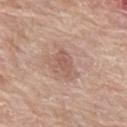| key | value |
|---|---|
| notes | catalogued during a skin exam; not biopsied |
| patient | female, aged approximately 60 |
| lesion diameter | about 3.5 mm |
| automated lesion analysis | a symmetry-axis asymmetry near 0.35; a mean CIELAB color near L≈57 a*≈20 b*≈26, a lesion–skin lightness drop of about 8, and a lesion-to-skin contrast of about 5.5 (normalized; higher = more distinct); a color-variation rating of about 2.5/10; a nevus-likeness score of about 0/100 and a detector confidence of about 100 out of 100 that the crop contains a lesion |
| body site | the leg |
| illumination | white-light |
| acquisition | ~15 mm crop, total-body skin-cancer survey |